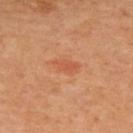{"biopsy_status": "not biopsied; imaged during a skin examination", "lighting": "cross-polarized", "site": "back", "patient": {"sex": "female", "age_approx": 65}, "image": {"source": "total-body photography crop", "field_of_view_mm": 15}}